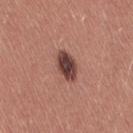Q: Illumination type?
A: white-light
Q: How was this image acquired?
A: total-body-photography crop, ~15 mm field of view
Q: Lesion size?
A: about 4 mm
Q: What are the patient's age and sex?
A: female, aged approximately 35
Q: Where on the body is the lesion?
A: the left thigh